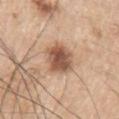{"biopsy_status": "not biopsied; imaged during a skin examination", "image": {"source": "total-body photography crop", "field_of_view_mm": 15}, "lighting": "white-light", "patient": {"sex": "male", "age_approx": 70}, "lesion_size": {"long_diameter_mm_approx": 4.5}, "site": "left upper arm"}